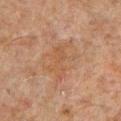| field | value |
|---|---|
| anatomic site | the chest |
| patient | male, aged 58–62 |
| lighting | cross-polarized |
| image | total-body-photography crop, ~15 mm field of view |